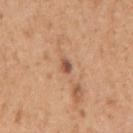<case>
  <biopsy_status>not biopsied; imaged during a skin examination</biopsy_status>
  <site>right upper arm</site>
  <image>
    <source>total-body photography crop</source>
    <field_of_view_mm>15</field_of_view_mm>
  </image>
  <patient>
    <sex>female</sex>
    <age_approx>40</age_approx>
  </patient>
</case>